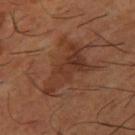Impression: Captured during whole-body skin photography for melanoma surveillance; the lesion was not biopsied. Background: A 15 mm close-up extracted from a 3D total-body photography capture. Located on the right thigh. The lesion-visualizer software estimated a border-irregularity index near 7.5/10, internal color variation of about 4 on a 0–10 scale, and a peripheral color-asymmetry measure near 1.5. The analysis additionally found an automated nevus-likeness rating near 0 out of 100 and a lesion-detection confidence of about 100/100. Measured at roughly 7 mm in maximum diameter. A male patient, about 65 years old.